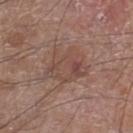This lesion was catalogued during total-body skin photography and was not selected for biopsy. About 6 mm across. Captured under white-light illumination. A male patient, aged around 60. The lesion is on the right lower leg. Cropped from a total-body skin-imaging series; the visible field is about 15 mm.